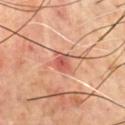Impression: This lesion was catalogued during total-body skin photography and was not selected for biopsy. Acquisition and patient details: This is a cross-polarized tile. About 2.5 mm across. Located on the chest. The patient is a male aged 68 to 72. Cropped from a whole-body photographic skin survey; the tile spans about 15 mm.